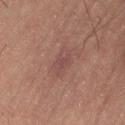Case summary:
- workup · catalogued during a skin exam; not biopsied
- illumination · cross-polarized illumination
- image source · total-body-photography crop, ~15 mm field of view
- anatomic site · the lower back
- subject · male, aged 48–52
- size · about 3.5 mm